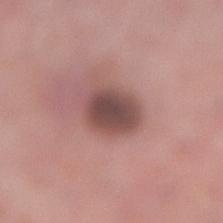<case>
  <biopsy_status>not biopsied; imaged during a skin examination</biopsy_status>
  <lesion_size>
    <long_diameter_mm_approx>4.5</long_diameter_mm_approx>
  </lesion_size>
  <image>
    <source>total-body photography crop</source>
    <field_of_view_mm>15</field_of_view_mm>
  </image>
  <site>leg</site>
  <patient>
    <sex>female</sex>
    <age_approx>40</age_approx>
  </patient>
</case>